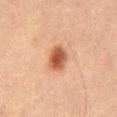Assessment: Captured during whole-body skin photography for melanoma surveillance; the lesion was not biopsied. Acquisition and patient details: Captured under cross-polarized illumination. The recorded lesion diameter is about 3 mm. The lesion is on the mid back. A region of skin cropped from a whole-body photographic capture, roughly 15 mm wide. A male subject, aged approximately 65.